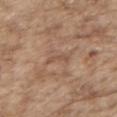biopsy_status: not biopsied; imaged during a skin examination